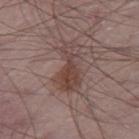Impression:
Part of a total-body skin-imaging series; this lesion was reviewed on a skin check and was not flagged for biopsy.
Acquisition and patient details:
This is a white-light tile. An algorithmic analysis of the crop reported roughly 8 lightness units darker than nearby skin and a normalized lesion–skin contrast near 7. The analysis additionally found an automated nevus-likeness rating near 70 out of 100 and a detector confidence of about 100 out of 100 that the crop contains a lesion. From the right thigh. A male subject aged around 65. Longest diameter approximately 5.5 mm. A region of skin cropped from a whole-body photographic capture, roughly 15 mm wide.